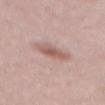workup: catalogued during a skin exam; not biopsied | patient: male, aged approximately 60 | anatomic site: the chest | image source: ~15 mm crop, total-body skin-cancer survey.